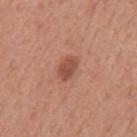This lesion was catalogued during total-body skin photography and was not selected for biopsy. A roughly 15 mm field-of-view crop from a total-body skin photograph. The total-body-photography lesion software estimated an area of roughly 4.5 mm² and an eccentricity of roughly 0.75. And it measured a mean CIELAB color near L≈50 a*≈25 b*≈28 and roughly 11 lightness units darker than nearby skin. A male patient about 55 years old. Captured under white-light illumination. The lesion is located on the mid back.